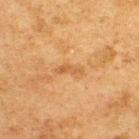Clinical impression: Part of a total-body skin-imaging series; this lesion was reviewed on a skin check and was not flagged for biopsy. Image and clinical context: The lesion is located on the upper back. Measured at roughly 3 mm in maximum diameter. The patient is a male about 75 years old. A lesion tile, about 15 mm wide, cut from a 3D total-body photograph.